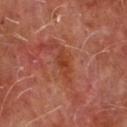site: chest
patient:
  sex: male
  age_approx: 65
automated_metrics:
  vs_skin_darker_L: 7.0
  vs_skin_contrast_norm: 7.0
  border_irregularity_0_10: 3.0
  color_variation_0_10: 2.0
  peripheral_color_asymmetry: 0.5
  lesion_detection_confidence_0_100: 100
image:
  source: total-body photography crop
  field_of_view_mm: 15
lighting: cross-polarized
lesion_size:
  long_diameter_mm_approx: 3.5